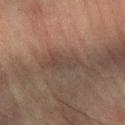No biopsy was performed on this lesion — it was imaged during a full skin examination and was not determined to be concerning. A male subject in their 70s. The lesion's longest dimension is about 4 mm. On the left forearm. This image is a 15 mm lesion crop taken from a total-body photograph.